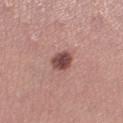The lesion was tiled from a total-body skin photograph and was not biopsied. Measured at roughly 3 mm in maximum diameter. Captured under white-light illumination. The subject is a female in their mid- to late 20s. A 15 mm close-up tile from a total-body photography series done for melanoma screening. The lesion is on the left lower leg.